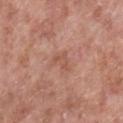<case>
  <lesion_size>
    <long_diameter_mm_approx>3.0</long_diameter_mm_approx>
  </lesion_size>
  <automated_metrics>
    <vs_skin_darker_L>6.0</vs_skin_darker_L>
    <border_irregularity_0_10>4.5</border_irregularity_0_10>
    <color_variation_0_10>1.5</color_variation_0_10>
    <peripheral_color_asymmetry>0.5</peripheral_color_asymmetry>
  </automated_metrics>
  <site>leg</site>
  <image>
    <source>total-body photography crop</source>
    <field_of_view_mm>15</field_of_view_mm>
  </image>
  <lighting>white-light</lighting>
  <patient>
    <sex>male</sex>
    <age_approx>55</age_approx>
  </patient>
</case>